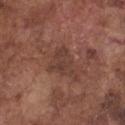workup: no biopsy performed (imaged during a skin exam) | subject: male, aged 73 to 77 | illumination: white-light | anatomic site: the chest | diameter: about 4 mm | automated metrics: an area of roughly 7 mm², an outline eccentricity of about 0.65 (0 = round, 1 = elongated), and a symmetry-axis asymmetry near 0.45; a mean CIELAB color near L≈39 a*≈20 b*≈24, about 6 CIELAB-L* units darker than the surrounding skin, and a lesion-to-skin contrast of about 5.5 (normalized; higher = more distinct); a border-irregularity rating of about 5/10, a color-variation rating of about 2.5/10, and radial color variation of about 1; an automated nevus-likeness rating near 0 out of 100 and a lesion-detection confidence of about 100/100 | acquisition: ~15 mm crop, total-body skin-cancer survey.